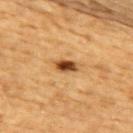lighting: cross-polarized
image source: ~15 mm crop, total-body skin-cancer survey
diameter: ≈3 mm
anatomic site: the upper back
patient: male, aged around 85 or older